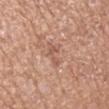The lesion is on the arm.
A 15 mm close-up tile from a total-body photography series done for melanoma screening.
An algorithmic analysis of the crop reported a lesion color around L≈56 a*≈22 b*≈29 in CIELAB, roughly 8 lightness units darker than nearby skin, and a normalized lesion–skin contrast near 5. And it measured a border-irregularity rating of about 6/10, a color-variation rating of about 0.5/10, and radial color variation of about 0.5.
A male subject roughly 75 years of age.